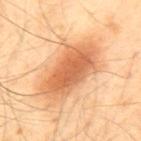Q: Was this lesion biopsied?
A: total-body-photography surveillance lesion; no biopsy
Q: What is the imaging modality?
A: ~15 mm crop, total-body skin-cancer survey
Q: What are the patient's age and sex?
A: male, aged approximately 40
Q: What did automated image analysis measure?
A: a footprint of about 24 mm²; a within-lesion color-variation index near 5/10 and radial color variation of about 1.5
Q: What lighting was used for the tile?
A: cross-polarized illumination
Q: Where on the body is the lesion?
A: the back
Q: How large is the lesion?
A: about 7.5 mm